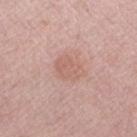Q: What lighting was used for the tile?
A: white-light illumination
Q: What kind of image is this?
A: ~15 mm tile from a whole-body skin photo
Q: Automated lesion metrics?
A: a lesion color around L≈61 a*≈21 b*≈26 in CIELAB, about 7 CIELAB-L* units darker than the surrounding skin, and a normalized border contrast of about 5; a border-irregularity index near 2.5/10, a color-variation rating of about 2/10, and a peripheral color-asymmetry measure near 0.5; a nevus-likeness score of about 0/100
Q: Patient demographics?
A: male, in their 60s
Q: What is the lesion's diameter?
A: ~3.5 mm (longest diameter)
Q: Where on the body is the lesion?
A: the right forearm